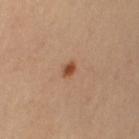Notes:
* body site · the left upper arm
* subject · female, in their mid- to late 30s
* imaging modality · ~15 mm tile from a whole-body skin photo